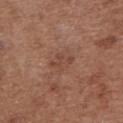| key | value |
|---|---|
| notes | no biopsy performed (imaged during a skin exam) |
| lesion diameter | ≈3 mm |
| location | the upper back |
| patient | female, aged 73 to 77 |
| image source | ~15 mm crop, total-body skin-cancer survey |